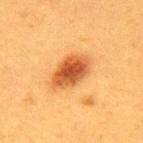Notes:
• follow-up — no biopsy performed (imaged during a skin exam)
• imaging modality — ~15 mm crop, total-body skin-cancer survey
• automated metrics — an area of roughly 12 mm², a shape eccentricity near 0.8, and a symmetry-axis asymmetry near 0.15; an average lesion color of about L≈47 a*≈26 b*≈40 (CIELAB), a lesion–skin lightness drop of about 14, and a normalized border contrast of about 10; lesion-presence confidence of about 100/100
• location — the back
• subject — female, roughly 40 years of age
• lesion diameter — ~5 mm (longest diameter)
• tile lighting — cross-polarized illumination